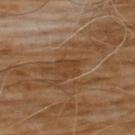No biopsy was performed on this lesion — it was imaged during a full skin examination and was not determined to be concerning. A male patient, about 60 years old. The lesion-visualizer software estimated an area of roughly 5 mm² and an eccentricity of roughly 0.65. The software also gave a border-irregularity rating of about 3/10 and a within-lesion color-variation index near 2.5/10. Captured under cross-polarized illumination. Cropped from a total-body skin-imaging series; the visible field is about 15 mm. The lesion's longest dimension is about 2.5 mm. The lesion is located on the chest.